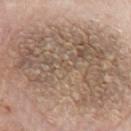Q: Was a biopsy performed?
A: total-body-photography surveillance lesion; no biopsy
Q: Who is the patient?
A: male, aged 73–77
Q: Lesion location?
A: the left upper arm
Q: Lesion size?
A: ≈14.5 mm
Q: What is the imaging modality?
A: total-body-photography crop, ~15 mm field of view